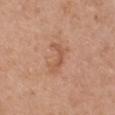| field | value |
|---|---|
| workup | catalogued during a skin exam; not biopsied |
| anatomic site | the chest |
| lesion diameter | ≈3.5 mm |
| automated lesion analysis | a footprint of about 5.5 mm² and an eccentricity of roughly 0.85; border irregularity of about 6.5 on a 0–10 scale, a within-lesion color-variation index near 1.5/10, and peripheral color asymmetry of about 0; an automated nevus-likeness rating near 0 out of 100 |
| patient | female, about 40 years old |
| lighting | white-light |
| imaging modality | ~15 mm crop, total-body skin-cancer survey |